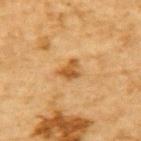Imaged during a routine full-body skin examination; the lesion was not biopsied and no histopathology is available. Measured at roughly 3 mm in maximum diameter. The tile uses cross-polarized illumination. On the upper back. A roughly 15 mm field-of-view crop from a total-body skin photograph. A male patient, aged around 85 or older. Automated image analysis of the tile measured roughly 10 lightness units darker than nearby skin and a normalized lesion–skin contrast near 8. The analysis additionally found border irregularity of about 3.5 on a 0–10 scale, a within-lesion color-variation index near 3.5/10, and a peripheral color-asymmetry measure near 1.